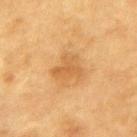Recorded during total-body skin imaging; not selected for excision or biopsy. Cropped from a total-body skin-imaging series; the visible field is about 15 mm. The lesion's longest dimension is about 4 mm. A male subject, aged 83 or older. The tile uses cross-polarized illumination. An algorithmic analysis of the crop reported a border-irregularity rating of about 4.5/10, internal color variation of about 3 on a 0–10 scale, and a peripheral color-asymmetry measure near 1. From the back.